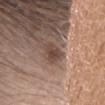Q: Is there a histopathology result?
A: imaged on a skin check; not biopsied
Q: Lesion location?
A: the head or neck
Q: What is the imaging modality?
A: ~15 mm crop, total-body skin-cancer survey
Q: What are the patient's age and sex?
A: female, about 35 years old